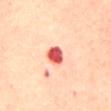Clinical impression: Imaged during a routine full-body skin examination; the lesion was not biopsied and no histopathology is available. Background: Automated tile analysis of the lesion measured an eccentricity of roughly 0.7 and a shape-asymmetry score of about 0.2 (0 = symmetric). A female patient aged approximately 45. A region of skin cropped from a whole-body photographic capture, roughly 15 mm wide. The lesion's longest dimension is about 3 mm. The lesion is on the mid back. Captured under cross-polarized illumination.